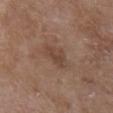No biopsy was performed on this lesion — it was imaged during a full skin examination and was not determined to be concerning.
A female patient, roughly 70 years of age.
A lesion tile, about 15 mm wide, cut from a 3D total-body photograph.
About 4 mm across.
This is a white-light tile.
The lesion is located on the upper back.